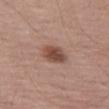Q: What is the imaging modality?
A: ~15 mm crop, total-body skin-cancer survey
Q: What are the patient's age and sex?
A: male, approximately 70 years of age
Q: What did automated image analysis measure?
A: two-axis asymmetry of about 0.15; a border-irregularity rating of about 1.5/10; an automated nevus-likeness rating near 95 out of 100
Q: How large is the lesion?
A: ~3 mm (longest diameter)
Q: Lesion location?
A: the left thigh